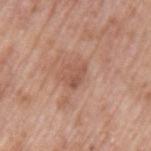notes=no biopsy performed (imaged during a skin exam); TBP lesion metrics=a border-irregularity rating of about 4/10; anatomic site=the left upper arm; imaging modality=total-body-photography crop, ~15 mm field of view; subject=male, aged 68–72; lighting=white-light illumination.